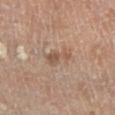- subject: male, aged 68 to 72
- image source: ~15 mm tile from a whole-body skin photo
- lesion size: about 3.5 mm
- lighting: cross-polarized illumination
- anatomic site: the left lower leg